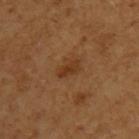follow-up — imaged on a skin check; not biopsied
image source — total-body-photography crop, ~15 mm field of view
body site — the arm
patient — female, in their mid-50s
tile lighting — cross-polarized